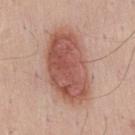<lesion>
  <biopsy_status>not biopsied; imaged during a skin examination</biopsy_status>
  <image>
    <source>total-body photography crop</source>
    <field_of_view_mm>15</field_of_view_mm>
  </image>
  <patient>
    <sex>male</sex>
    <age_approx>35</age_approx>
  </patient>
  <site>mid back</site>
</lesion>